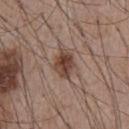<tbp_lesion>
  <biopsy_status>not biopsied; imaged during a skin examination</biopsy_status>
  <site>chest</site>
  <patient>
    <sex>male</sex>
    <age_approx>55</age_approx>
  </patient>
  <image>
    <source>total-body photography crop</source>
    <field_of_view_mm>15</field_of_view_mm>
  </image>
  <lighting>white-light</lighting>
  <lesion_size>
    <long_diameter_mm_approx>3.5</long_diameter_mm_approx>
  </lesion_size>
</tbp_lesion>